Cropped from a whole-body photographic skin survey; the tile spans about 15 mm. Located on the head or neck. The patient is a female approximately 75 years of age. The lesion-visualizer software estimated two-axis asymmetry of about 0.45. This is a cross-polarized tile. Approximately 1 mm at its widest.
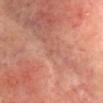pathology — an actinic keratosis (borderline).